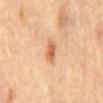workup=no biopsy performed (imaged during a skin exam); tile lighting=cross-polarized illumination; imaging modality=~15 mm tile from a whole-body skin photo; body site=the back; automated metrics=a nevus-likeness score of about 55/100 and a lesion-detection confidence of about 100/100; subject=male, roughly 85 years of age or older; size=≈2.5 mm.